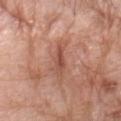biopsy status: imaged on a skin check; not biopsied
site: the arm
tile lighting: white-light illumination
size: about 3.5 mm
patient: male, aged approximately 60
image source: 15 mm crop, total-body photography
automated lesion analysis: an average lesion color of about L≈51 a*≈25 b*≈29 (CIELAB), about 9 CIELAB-L* units darker than the surrounding skin, and a normalized lesion–skin contrast near 6.5; a border-irregularity rating of about 4/10 and internal color variation of about 2 on a 0–10 scale